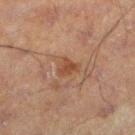subject = male, about 60 years old
lesion size = about 2.5 mm
location = the left lower leg
lighting = cross-polarized
image = 15 mm crop, total-body photography
image-analysis metrics = a lesion color around L≈35 a*≈17 b*≈25 in CIELAB, about 6 CIELAB-L* units darker than the surrounding skin, and a normalized lesion–skin contrast near 6.5; a border-irregularity rating of about 4/10, a within-lesion color-variation index near 1.5/10, and a peripheral color-asymmetry measure near 0.5; a classifier nevus-likeness of about 0/100